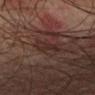Imaged during a routine full-body skin examination; the lesion was not biopsied and no histopathology is available.
The tile uses cross-polarized illumination.
Located on the arm.
A male subject, aged 73 to 77.
This image is a 15 mm lesion crop taken from a total-body photograph.